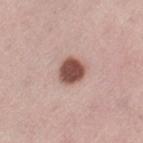A female subject, aged 43 to 47. A 15 mm close-up tile from a total-body photography series done for melanoma screening. This is a white-light tile. On the left thigh.